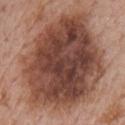subject — male, aged approximately 75 | lesion diameter — ~13.5 mm (longest diameter) | site — the chest | image — ~15 mm crop, total-body skin-cancer survey.